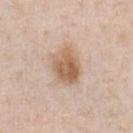  biopsy_status: not biopsied; imaged during a skin examination
  patient:
    sex: male
    age_approx: 40
  automated_metrics:
    area_mm2_approx: 13.0
    eccentricity: 0.65
    shape_asymmetry: 0.2
    color_variation_0_10: 5.5
    lesion_detection_confidence_0_100: 100
  image:
    source: total-body photography crop
    field_of_view_mm: 15
  lighting: white-light
  lesion_size:
    long_diameter_mm_approx: 4.5
  site: chest